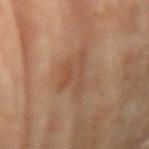| field | value |
|---|---|
| illumination | cross-polarized illumination |
| TBP lesion metrics | a lesion color around L≈42 a*≈16 b*≈27 in CIELAB and a normalized lesion–skin contrast near 5; border irregularity of about 6 on a 0–10 scale, a color-variation rating of about 3/10, and radial color variation of about 1 |
| site | the right forearm |
| acquisition | ~15 mm tile from a whole-body skin photo |
| patient | female, aged around 80 |
| lesion diameter | ~5 mm (longest diameter) |